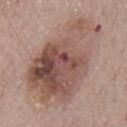{"biopsy_status": "not biopsied; imaged during a skin examination", "lesion_size": {"long_diameter_mm_approx": 11.5}, "lighting": "white-light", "patient": {"sex": "male", "age_approx": 75}, "image": {"source": "total-body photography crop", "field_of_view_mm": 15}, "site": "back"}